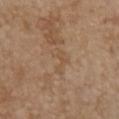imaging modality — 15 mm crop, total-body photography; location — the chest; subject — female, aged approximately 65.